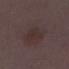  biopsy_status: not biopsied; imaged during a skin examination
  image:
    source: total-body photography crop
    field_of_view_mm: 15
  patient:
    sex: female
    age_approx: 30
  site: left lower leg
  lighting: white-light
  lesion_size:
    long_diameter_mm_approx: 3.5